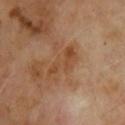<tbp_lesion>
<biopsy_status>not biopsied; imaged during a skin examination</biopsy_status>
<automated_metrics>
  <cielab_L>44</cielab_L>
  <cielab_a>20</cielab_a>
  <cielab_b>33</cielab_b>
  <vs_skin_darker_L>6.0</vs_skin_darker_L>
  <vs_skin_contrast_norm>6.0</vs_skin_contrast_norm>
  <nevus_likeness_0_100>0</nevus_likeness_0_100>
  <lesion_detection_confidence_0_100>100</lesion_detection_confidence_0_100>
</automated_metrics>
<image>
  <source>total-body photography crop</source>
  <field_of_view_mm>15</field_of_view_mm>
</image>
<site>front of the torso</site>
<lighting>cross-polarized</lighting>
<patient>
  <sex>male</sex>
  <age_approx>65</age_approx>
</patient>
<lesion_size>
  <long_diameter_mm_approx>5.0</long_diameter_mm_approx>
</lesion_size>
</tbp_lesion>